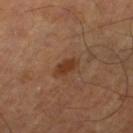Clinical impression:
Imaged during a routine full-body skin examination; the lesion was not biopsied and no histopathology is available.
Background:
The lesion is located on the right thigh. The total-body-photography lesion software estimated a lesion color around L≈35 a*≈21 b*≈31 in CIELAB, a lesion–skin lightness drop of about 9, and a normalized border contrast of about 8.5. It also reported a classifier nevus-likeness of about 85/100. The patient is roughly 65 years of age. Longest diameter approximately 3 mm. A region of skin cropped from a whole-body photographic capture, roughly 15 mm wide. Imaged with cross-polarized lighting.